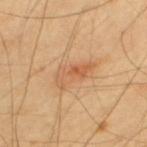{
  "biopsy_status": "not biopsied; imaged during a skin examination",
  "lighting": "cross-polarized",
  "automated_metrics": {
    "cielab_L": 58,
    "cielab_a": 23,
    "cielab_b": 37,
    "vs_skin_darker_L": 9.0,
    "vs_skin_contrast_norm": 6.0,
    "border_irregularity_0_10": 7.5,
    "color_variation_0_10": 2.0,
    "peripheral_color_asymmetry": 0.5
  },
  "lesion_size": {
    "long_diameter_mm_approx": 4.5
  },
  "site": "upper back",
  "patient": {
    "sex": "male",
    "age_approx": 65
  },
  "image": {
    "source": "total-body photography crop",
    "field_of_view_mm": 15
  }
}